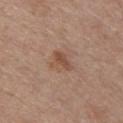Case summary:
– biopsy status — imaged on a skin check; not biopsied
– lighting — white-light illumination
– automated metrics — an average lesion color of about L≈50 a*≈19 b*≈29 (CIELAB), roughly 8 lightness units darker than nearby skin, and a normalized border contrast of about 6.5
– imaging modality — 15 mm crop, total-body photography
– lesion size — ≈3 mm
– location — the chest
– subject — male, in their 30s